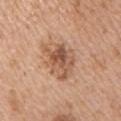Part of a total-body skin-imaging series; this lesion was reviewed on a skin check and was not flagged for biopsy. From the chest. Longest diameter approximately 5.5 mm. The patient is a female about 45 years old. The tile uses white-light illumination. A roughly 15 mm field-of-view crop from a total-body skin photograph.